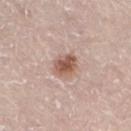* follow-up: catalogued during a skin exam; not biopsied
* tile lighting: white-light illumination
* patient: male, aged 78 to 82
* location: the leg
* acquisition: ~15 mm tile from a whole-body skin photo